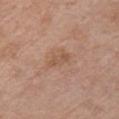Q: Was a biopsy performed?
A: imaged on a skin check; not biopsied